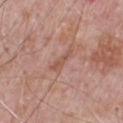Clinical impression: This lesion was catalogued during total-body skin photography and was not selected for biopsy.